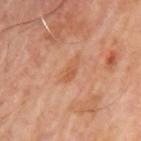Q: Is there a histopathology result?
A: imaged on a skin check; not biopsied
Q: What is the anatomic site?
A: the left upper arm
Q: Illumination type?
A: cross-polarized illumination
Q: Who is the patient?
A: male, in their mid-60s
Q: How was this image acquired?
A: ~15 mm tile from a whole-body skin photo
Q: How large is the lesion?
A: ≈3.5 mm